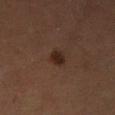biopsy status = total-body-photography surveillance lesion; no biopsy
automated lesion analysis = a lesion area of about 3.5 mm², an eccentricity of roughly 0.7, and a symmetry-axis asymmetry near 0.25
site = the leg
imaging modality = ~15 mm crop, total-body skin-cancer survey
subject = female, aged 53–57
lesion diameter = ~2.5 mm (longest diameter)